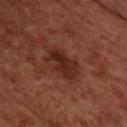Part of a total-body skin-imaging series; this lesion was reviewed on a skin check and was not flagged for biopsy.
The lesion is on the right forearm.
Captured under cross-polarized illumination.
Automated tile analysis of the lesion measured a footprint of about 8 mm², a shape eccentricity near 0.85, and two-axis asymmetry of about 0.25. The software also gave a within-lesion color-variation index near 4/10. It also reported a detector confidence of about 100 out of 100 that the crop contains a lesion.
A female patient roughly 65 years of age.
Approximately 4.5 mm at its widest.
A region of skin cropped from a whole-body photographic capture, roughly 15 mm wide.